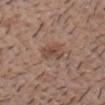biopsy status: imaged on a skin check; not biopsied | site: the head or neck | image source: ~15 mm tile from a whole-body skin photo | subject: male, aged 48 to 52.